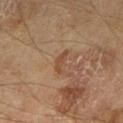Captured under cross-polarized illumination. Measured at roughly 3 mm in maximum diameter. From the left thigh. A 15 mm close-up extracted from a 3D total-body photography capture. A male patient aged 68–72.